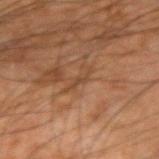notes = imaged on a skin check; not biopsied
size = ~3.5 mm (longest diameter)
image source = 15 mm crop, total-body photography
lighting = cross-polarized
subject = male, approximately 65 years of age
anatomic site = the right forearm